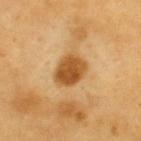Findings:
– biopsy status — total-body-photography surveillance lesion; no biopsy
– image — ~15 mm crop, total-body skin-cancer survey
– location — the upper back
– patient — male, roughly 60 years of age